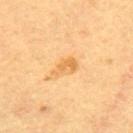Case summary:
• diameter — ~3 mm (longest diameter)
• image — 15 mm crop, total-body photography
• location — the mid back
• subject — female, aged 58–62
• TBP lesion metrics — an area of roughly 4 mm², a shape eccentricity near 0.85, and a symmetry-axis asymmetry near 0.45; a lesion–skin lightness drop of about 8 and a normalized border contrast of about 6.5; internal color variation of about 1 on a 0–10 scale and a peripheral color-asymmetry measure near 0.5
• tile lighting — cross-polarized